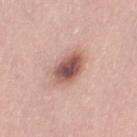notes — total-body-photography surveillance lesion; no biopsy | site — the leg | diameter — ~4 mm (longest diameter) | patient — female, in their mid- to late 40s | lighting — white-light | acquisition — ~15 mm crop, total-body skin-cancer survey | TBP lesion metrics — a lesion area of about 10 mm² and a shape-asymmetry score of about 0.15 (0 = symmetric); a lesion color around L≈55 a*≈22 b*≈24 in CIELAB and roughly 16 lightness units darker than nearby skin; border irregularity of about 1.5 on a 0–10 scale; an automated nevus-likeness rating near 70 out of 100 and a lesion-detection confidence of about 100/100.